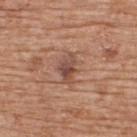Captured during whole-body skin photography for melanoma surveillance; the lesion was not biopsied.
A roughly 15 mm field-of-view crop from a total-body skin photograph.
A male patient, aged around 60.
On the upper back.